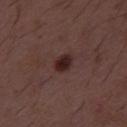Findings:
• biopsy status · imaged on a skin check; not biopsied
• tile lighting · white-light illumination
• imaging modality · total-body-photography crop, ~15 mm field of view
• patient · male, aged 48 to 52
• size · ≈2.5 mm
• image-analysis metrics · border irregularity of about 1.5 on a 0–10 scale, a color-variation rating of about 2.5/10, and radial color variation of about 0.5